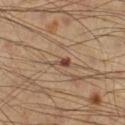<tbp_lesion>
<biopsy_status>not biopsied; imaged during a skin examination</biopsy_status>
<lesion_size>
  <long_diameter_mm_approx>2.5</long_diameter_mm_approx>
</lesion_size>
<automated_metrics>
  <cielab_L>47</cielab_L>
  <cielab_a>19</cielab_a>
  <cielab_b>28</cielab_b>
  <vs_skin_darker_L>11.0</vs_skin_darker_L>
  <vs_skin_contrast_norm>8.0</vs_skin_contrast_norm>
  <border_irregularity_0_10>4.0</border_irregularity_0_10>
  <color_variation_0_10>1.0</color_variation_0_10>
  <peripheral_color_asymmetry>0.0</peripheral_color_asymmetry>
  <nevus_likeness_0_100>75</nevus_likeness_0_100>
  <lesion_detection_confidence_0_100>100</lesion_detection_confidence_0_100>
</automated_metrics>
<lighting>cross-polarized</lighting>
<patient>
  <sex>male</sex>
  <age_approx>40</age_approx>
</patient>
<site>right lower leg</site>
<image>
  <source>total-body photography crop</source>
  <field_of_view_mm>15</field_of_view_mm>
</image>
</tbp_lesion>